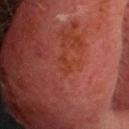Case summary:
• notes — catalogued during a skin exam; not biopsied
• anatomic site — the head or neck
• lighting — cross-polarized illumination
• patient — male, approximately 60 years of age
• size — ~3 mm (longest diameter)
• acquisition — ~15 mm tile from a whole-body skin photo
• automated metrics — a footprint of about 2.5 mm², an outline eccentricity of about 0.9 (0 = round, 1 = elongated), and two-axis asymmetry of about 0.45; a border-irregularity index near 5.5/10 and a color-variation rating of about 0/10; a classifier nevus-likeness of about 0/100